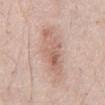{
  "biopsy_status": "not biopsied; imaged during a skin examination"
}